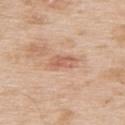Case summary:
- notes — imaged on a skin check; not biopsied
- image — 15 mm crop, total-body photography
- lesion size — about 3 mm
- location — the upper back
- tile lighting — white-light illumination
- patient — male, in their mid-60s